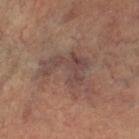* follow-up — total-body-photography surveillance lesion; no biopsy
* location — the right leg
* patient — female, roughly 45 years of age
* image source — ~15 mm crop, total-body skin-cancer survey
* lighting — cross-polarized illumination
* lesion diameter — about 7 mm